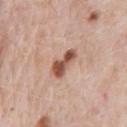Captured during whole-body skin photography for melanoma surveillance; the lesion was not biopsied. On the chest. A 15 mm close-up tile from a total-body photography series done for melanoma screening. Imaged with white-light lighting. A male patient, in their 80s.